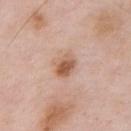The lesion was photographed on a routine skin check and not biopsied; there is no pathology result.
From the chest.
A male patient aged 53 to 57.
A 15 mm close-up tile from a total-body photography series done for melanoma screening.